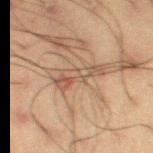Q: Is there a histopathology result?
A: imaged on a skin check; not biopsied
Q: How was this image acquired?
A: ~15 mm tile from a whole-body skin photo
Q: Lesion size?
A: about 5 mm
Q: Patient demographics?
A: male, aged 58 to 62
Q: What is the anatomic site?
A: the left thigh
Q: What did automated image analysis measure?
A: about 7 CIELAB-L* units darker than the surrounding skin and a lesion-to-skin contrast of about 6 (normalized; higher = more distinct); border irregularity of about 5.5 on a 0–10 scale, a color-variation rating of about 5/10, and radial color variation of about 1.5; a nevus-likeness score of about 0/100 and a lesion-detection confidence of about 70/100
Q: How was the tile lit?
A: cross-polarized